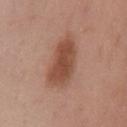follow-up: no biopsy performed (imaged during a skin exam)
lighting: white-light
lesion size: ~7 mm (longest diameter)
body site: the chest
image: ~15 mm tile from a whole-body skin photo
patient: male, roughly 55 years of age